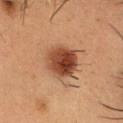A region of skin cropped from a whole-body photographic capture, roughly 15 mm wide. The tile uses cross-polarized illumination. Longest diameter approximately 5 mm. On the head or neck. The subject is a male roughly 35 years of age.